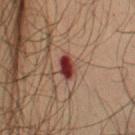workup: catalogued during a skin exam; not biopsied | site: the arm | automated metrics: a mean CIELAB color near L≈27 a*≈22 b*≈20 | subject: male, aged around 50 | image source: 15 mm crop, total-body photography.